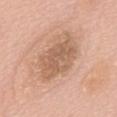biopsy status=imaged on a skin check; not biopsied | acquisition=total-body-photography crop, ~15 mm field of view | body site=the mid back | image-analysis metrics=an area of roughly 18 mm² and an eccentricity of roughly 0.8; a within-lesion color-variation index near 3.5/10; a classifier nevus-likeness of about 0/100 and lesion-presence confidence of about 100/100 | subject=female, about 60 years old | illumination=white-light | lesion diameter=~6 mm (longest diameter).